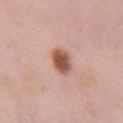Captured during whole-body skin photography for melanoma surveillance; the lesion was not biopsied.